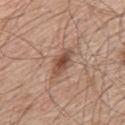biopsy status = total-body-photography surveillance lesion; no biopsy
patient = male, aged approximately 65
diameter = about 4.5 mm
acquisition = 15 mm crop, total-body photography
TBP lesion metrics = a footprint of about 7 mm², an eccentricity of roughly 0.85, and two-axis asymmetry of about 0.2; a lesion color around L≈51 a*≈19 b*≈28 in CIELAB, a lesion–skin lightness drop of about 11, and a normalized lesion–skin contrast near 8; an automated nevus-likeness rating near 85 out of 100
body site = the upper back
illumination = white-light